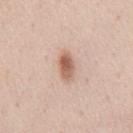A male patient roughly 45 years of age. A close-up tile cropped from a whole-body skin photograph, about 15 mm across. Automated tile analysis of the lesion measured a within-lesion color-variation index near 6/10 and peripheral color asymmetry of about 2. And it measured a classifier nevus-likeness of about 100/100 and lesion-presence confidence of about 100/100. The lesion is located on the chest. The tile uses white-light illumination.